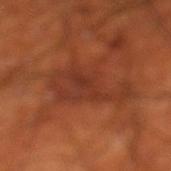Captured during whole-body skin photography for melanoma surveillance; the lesion was not biopsied. Approximately 8 mm at its widest. A region of skin cropped from a whole-body photographic capture, roughly 15 mm wide. The subject is a male aged approximately 70. Located on the left lower leg. This is a cross-polarized tile.